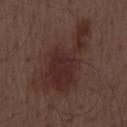{
  "lesion_size": {
    "long_diameter_mm_approx": 10.5
  },
  "site": "mid back",
  "image": {
    "source": "total-body photography crop",
    "field_of_view_mm": 15
  },
  "patient": {
    "sex": "male",
    "age_approx": 55
  }
}